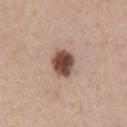No biopsy was performed on this lesion — it was imaged during a full skin examination and was not determined to be concerning.
The tile uses white-light illumination.
A female subject, about 45 years old.
Approximately 3.5 mm at its widest.
From the abdomen.
A 15 mm close-up extracted from a 3D total-body photography capture.